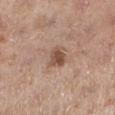{"biopsy_status": "not biopsied; imaged during a skin examination", "automated_metrics": {"cielab_L": 50, "cielab_a": 20, "cielab_b": 27, "vs_skin_darker_L": 12.0, "vs_skin_contrast_norm": 8.5, "border_irregularity_0_10": 2.0, "color_variation_0_10": 3.0, "peripheral_color_asymmetry": 1.0, "nevus_likeness_0_100": 35, "lesion_detection_confidence_0_100": 100}, "image": {"source": "total-body photography crop", "field_of_view_mm": 15}, "patient": {"sex": "female", "age_approx": 40}, "lighting": "white-light", "site": "left lower leg"}